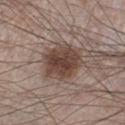* follow-up · imaged on a skin check; not biopsied
* subject · male, about 60 years old
* lighting · white-light illumination
* anatomic site · the right lower leg
* size · ≈5 mm
* imaging modality · ~15 mm tile from a whole-body skin photo